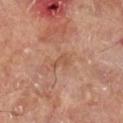Impression: Recorded during total-body skin imaging; not selected for excision or biopsy. Context: Measured at roughly 2.5 mm in maximum diameter. Captured under cross-polarized illumination. A 15 mm close-up extracted from a 3D total-body photography capture. From the right lower leg.